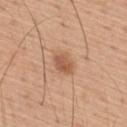The lesion was photographed on a routine skin check and not biopsied; there is no pathology result. Located on the upper back. This image is a 15 mm lesion crop taken from a total-body photograph. About 3 mm across. The subject is a male in their mid-50s.